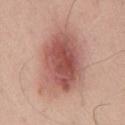Image and clinical context: The patient is a male aged 53–57. Approximately 8.5 mm at its widest. Located on the chest. This is a white-light tile. A lesion tile, about 15 mm wide, cut from a 3D total-body photograph. The total-body-photography lesion software estimated an outline eccentricity of about 0.75 (0 = round, 1 = elongated). The analysis additionally found roughly 14 lightness units darker than nearby skin and a normalized border contrast of about 9. It also reported border irregularity of about 2 on a 0–10 scale and a color-variation rating of about 7/10.